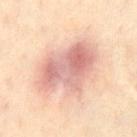biopsy status = imaged on a skin check; not biopsied | automated metrics = a lesion color around L≈67 a*≈23 b*≈25 in CIELAB and a lesion–skin lightness drop of about 12; a border-irregularity index near 3.5/10, a color-variation rating of about 6.5/10, and radial color variation of about 2 | diameter = ~7 mm (longest diameter) | patient = male, in their mid- to late 30s | anatomic site = the chest | tile lighting = cross-polarized | acquisition = 15 mm crop, total-body photography.